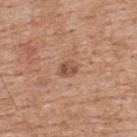Captured during whole-body skin photography for melanoma surveillance; the lesion was not biopsied. From the upper back. A male subject in their 60s. A 15 mm close-up extracted from a 3D total-body photography capture.